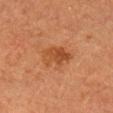Impression:
The lesion was tiled from a total-body skin photograph and was not biopsied.
Acquisition and patient details:
Measured at roughly 4 mm in maximum diameter. A lesion tile, about 15 mm wide, cut from a 3D total-body photograph. On the head or neck. Captured under cross-polarized illumination. Automated tile analysis of the lesion measured a border-irregularity index near 3.5/10, a color-variation rating of about 4/10, and radial color variation of about 1.5. The patient is a female in their 70s.